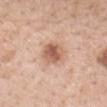<lesion>
<biopsy_status>not biopsied; imaged during a skin examination</biopsy_status>
<image>
  <source>total-body photography crop</source>
  <field_of_view_mm>15</field_of_view_mm>
</image>
<patient>
  <sex>female</sex>
  <age_approx>50</age_approx>
</patient>
<lighting>white-light</lighting>
<automated_metrics>
  <area_mm2_approx>8.0</area_mm2_approx>
  <eccentricity>0.55</eccentricity>
  <shape_asymmetry>0.15</shape_asymmetry>
  <border_irregularity_0_10>2.0</border_irregularity_0_10>
  <color_variation_0_10>5.0</color_variation_0_10>
  <peripheral_color_asymmetry>1.5</peripheral_color_asymmetry>
</automated_metrics>
<lesion_size>
  <long_diameter_mm_approx>3.5</long_diameter_mm_approx>
</lesion_size>
<site>mid back</site>
</lesion>